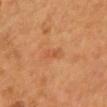| key | value |
|---|---|
| biopsy status | catalogued during a skin exam; not biopsied |
| lesion size | about 3 mm |
| anatomic site | the back |
| image | ~15 mm crop, total-body skin-cancer survey |
| TBP lesion metrics | a border-irregularity index near 3/10, a within-lesion color-variation index near 2/10, and peripheral color asymmetry of about 0.5 |
| lighting | cross-polarized illumination |
| subject | male, in their mid-50s |